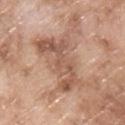Impression: The lesion was photographed on a routine skin check and not biopsied; there is no pathology result. Background: The recorded lesion diameter is about 9 mm. The lesion is on the upper back. A lesion tile, about 15 mm wide, cut from a 3D total-body photograph. The total-body-photography lesion software estimated a footprint of about 25 mm², a shape eccentricity near 0.85, and a symmetry-axis asymmetry near 0.45. And it measured a lesion color around L≈57 a*≈20 b*≈29 in CIELAB and a lesion-to-skin contrast of about 6.5 (normalized; higher = more distinct). And it measured a classifier nevus-likeness of about 0/100 and a detector confidence of about 55 out of 100 that the crop contains a lesion. Captured under white-light illumination. A male subject aged 63 to 67.